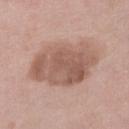Imaged during a routine full-body skin examination; the lesion was not biopsied and no histopathology is available. A roughly 15 mm field-of-view crop from a total-body skin photograph. The patient is a female roughly 40 years of age. From the right lower leg.